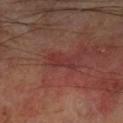Part of a total-body skin-imaging series; this lesion was reviewed on a skin check and was not flagged for biopsy. Longest diameter approximately 3.5 mm. The patient is a male aged approximately 70. A 15 mm close-up extracted from a 3D total-body photography capture. Captured under cross-polarized illumination. The lesion is located on the left lower leg.